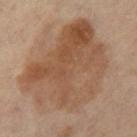Q: How was this image acquired?
A: ~15 mm tile from a whole-body skin photo
Q: What did automated image analysis measure?
A: a lesion area of about 65 mm² and a shape-asymmetry score of about 0.25 (0 = symmetric); a lesion color around L≈49 a*≈18 b*≈30 in CIELAB and about 9 CIELAB-L* units darker than the surrounding skin; border irregularity of about 3.5 on a 0–10 scale, a color-variation rating of about 6.5/10, and a peripheral color-asymmetry measure near 2.5; a classifier nevus-likeness of about 10/100 and lesion-presence confidence of about 100/100
Q: What are the patient's age and sex?
A: female, aged approximately 65
Q: Where on the body is the lesion?
A: the left leg